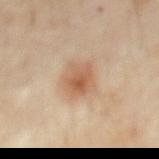No biopsy was performed on this lesion — it was imaged during a full skin examination and was not determined to be concerning.
About 4 mm across.
Captured under cross-polarized illumination.
Cropped from a total-body skin-imaging series; the visible field is about 15 mm.
On the chest.
A male subject, aged around 60.